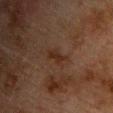This lesion was catalogued during total-body skin photography and was not selected for biopsy.